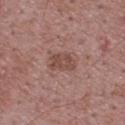Impression:
This lesion was catalogued during total-body skin photography and was not selected for biopsy.
Clinical summary:
The patient is a male aged around 70. A 15 mm crop from a total-body photograph taken for skin-cancer surveillance. The lesion is located on the upper back. The recorded lesion diameter is about 3.5 mm.